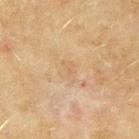The lesion was photographed on a routine skin check and not biopsied; there is no pathology result. A female subject, aged approximately 55. A region of skin cropped from a whole-body photographic capture, roughly 15 mm wide. Captured under cross-polarized illumination. Automated image analysis of the tile measured a color-variation rating of about 0/10. And it measured a nevus-likeness score of about 0/100 and a detector confidence of about 100 out of 100 that the crop contains a lesion. The lesion's longest dimension is about 1.5 mm. The lesion is on the left upper arm.